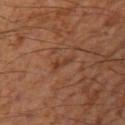Part of a total-body skin-imaging series; this lesion was reviewed on a skin check and was not flagged for biopsy. The lesion is on the leg. A male patient in their mid-50s. A region of skin cropped from a whole-body photographic capture, roughly 15 mm wide.